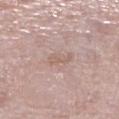- follow-up — catalogued during a skin exam; not biopsied
- patient — female, aged approximately 60
- location — the leg
- acquisition — 15 mm crop, total-body photography
- tile lighting — white-light illumination
- size — ≈3 mm